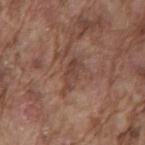Captured during whole-body skin photography for melanoma surveillance; the lesion was not biopsied. The lesion is located on the back. A lesion tile, about 15 mm wide, cut from a 3D total-body photograph. A male subject, roughly 75 years of age. The total-body-photography lesion software estimated a footprint of about 4.5 mm², an outline eccentricity of about 0.9 (0 = round, 1 = elongated), and a shape-asymmetry score of about 0.3 (0 = symmetric). It also reported an average lesion color of about L≈42 a*≈19 b*≈25 (CIELAB), a lesion–skin lightness drop of about 8, and a normalized lesion–skin contrast near 6.5. It also reported a border-irregularity index near 4.5/10, a within-lesion color-variation index near 2/10, and radial color variation of about 0.5. The software also gave an automated nevus-likeness rating near 0 out of 100. Captured under white-light illumination.